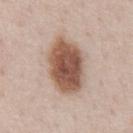The lesion was photographed on a routine skin check and not biopsied; there is no pathology result.